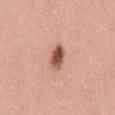  biopsy_status: not biopsied; imaged during a skin examination
  lighting: white-light
  image:
    source: total-body photography crop
    field_of_view_mm: 15
  site: abdomen
  patient:
    sex: male
    age_approx: 60
  lesion_size:
    long_diameter_mm_approx: 3.0